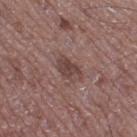On the right thigh. A 15 mm close-up tile from a total-body photography series done for melanoma screening. Approximately 3.5 mm at its widest. An algorithmic analysis of the crop reported an area of roughly 5 mm², a shape eccentricity near 0.8, and a shape-asymmetry score of about 0.4 (0 = symmetric). The software also gave roughly 9 lightness units darker than nearby skin and a normalized lesion–skin contrast near 7.5. And it measured a classifier nevus-likeness of about 5/100 and a lesion-detection confidence of about 100/100. A male subject in their 50s.